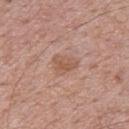Q: Is there a histopathology result?
A: no biopsy performed (imaged during a skin exam)
Q: What lighting was used for the tile?
A: white-light
Q: What did automated image analysis measure?
A: an average lesion color of about L≈55 a*≈21 b*≈29 (CIELAB), about 8 CIELAB-L* units darker than the surrounding skin, and a normalized lesion–skin contrast near 6
Q: Lesion location?
A: the upper back
Q: What is the imaging modality?
A: ~15 mm tile from a whole-body skin photo
Q: Who is the patient?
A: male, roughly 70 years of age
Q: How large is the lesion?
A: ≈3 mm